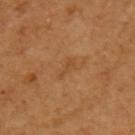Captured during whole-body skin photography for melanoma surveillance; the lesion was not biopsied. The lesion is on the arm. Automated tile analysis of the lesion measured an area of roughly 2.5 mm². And it measured a mean CIELAB color near L≈49 a*≈22 b*≈39, a lesion–skin lightness drop of about 6, and a normalized border contrast of about 4.5. It also reported border irregularity of about 4.5 on a 0–10 scale, a color-variation rating of about 0/10, and peripheral color asymmetry of about 0. Longest diameter approximately 3 mm. The patient is a female approximately 55 years of age. A lesion tile, about 15 mm wide, cut from a 3D total-body photograph.